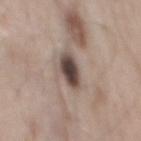notes: catalogued during a skin exam; not biopsied
lesion diameter: about 3.5 mm
subject: male, aged 53–57
location: the mid back
automated lesion analysis: a lesion area of about 7 mm² and a shape-asymmetry score of about 0.25 (0 = symmetric); a lesion color around L≈44 a*≈13 b*≈19 in CIELAB, about 18 CIELAB-L* units darker than the surrounding skin, and a normalized border contrast of about 13
image source: ~15 mm crop, total-body skin-cancer survey